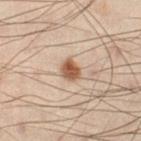<record>
  <biopsy_status>not biopsied; imaged during a skin examination</biopsy_status>
  <patient>
    <sex>male</sex>
    <age_approx>50</age_approx>
  </patient>
  <lighting>cross-polarized</lighting>
  <image>
    <source>total-body photography crop</source>
    <field_of_view_mm>15</field_of_view_mm>
  </image>
  <site>left lower leg</site>
  <lesion_size>
    <long_diameter_mm_approx>2.5</long_diameter_mm_approx>
  </lesion_size>
</record>